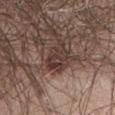Recorded during total-body skin imaging; not selected for excision or biopsy.
A region of skin cropped from a whole-body photographic capture, roughly 15 mm wide.
The lesion is located on the abdomen.
The recorded lesion diameter is about 4 mm.
The patient is a male aged 53 to 57.
Automated image analysis of the tile measured roughly 9 lightness units darker than nearby skin. The analysis additionally found a border-irregularity rating of about 3/10, internal color variation of about 5 on a 0–10 scale, and peripheral color asymmetry of about 1.5. The software also gave a nevus-likeness score of about 10/100 and a lesion-detection confidence of about 80/100.
Imaged with white-light lighting.